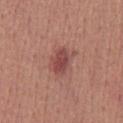Clinical impression: No biopsy was performed on this lesion — it was imaged during a full skin examination and was not determined to be concerning. Clinical summary: On the chest. A close-up tile cropped from a whole-body skin photograph, about 15 mm across. The lesion-visualizer software estimated a lesion color around L≈48 a*≈25 b*≈24 in CIELAB, roughly 10 lightness units darker than nearby skin, and a lesion-to-skin contrast of about 7.5 (normalized; higher = more distinct). Longest diameter approximately 4 mm. The tile uses white-light illumination. A male subject, aged around 45.